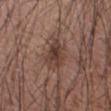Clinical impression: No biopsy was performed on this lesion — it was imaged during a full skin examination and was not determined to be concerning. Context: The lesion is located on the right upper arm. A male subject, about 65 years old. An algorithmic analysis of the crop reported a lesion area of about 7 mm² and a symmetry-axis asymmetry near 0.35. And it measured a border-irregularity rating of about 3/10, a color-variation rating of about 5/10, and a peripheral color-asymmetry measure near 1.5. A lesion tile, about 15 mm wide, cut from a 3D total-body photograph. About 3.5 mm across.